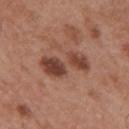Notes:
- workup · no biopsy performed (imaged during a skin exam)
- tile lighting · white-light
- image source · ~15 mm tile from a whole-body skin photo
- automated lesion analysis · a footprint of about 12 mm², a shape eccentricity near 0.9, and a shape-asymmetry score of about 0.4 (0 = symmetric); about 12 CIELAB-L* units darker than the surrounding skin and a lesion-to-skin contrast of about 9 (normalized; higher = more distinct); a classifier nevus-likeness of about 20/100 and a lesion-detection confidence of about 100/100
- subject · male, aged approximately 55
- lesion size · about 5.5 mm
- body site · the mid back